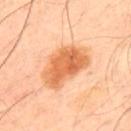workup = imaged on a skin check; not biopsied
image = total-body-photography crop, ~15 mm field of view
image-analysis metrics = an automated nevus-likeness rating near 100 out of 100 and a detector confidence of about 100 out of 100 that the crop contains a lesion
patient = male, aged 48 to 52
body site = the upper back